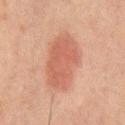A male patient aged 63–67. A 15 mm close-up extracted from a 3D total-body photography capture. Longest diameter approximately 6.5 mm. The lesion is on the back. Imaged with cross-polarized lighting.